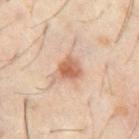{"biopsy_status": "not biopsied; imaged during a skin examination", "lighting": "cross-polarized", "lesion_size": {"long_diameter_mm_approx": 3.0}, "site": "abdomen", "automated_metrics": {"area_mm2_approx": 5.5, "eccentricity": 0.4, "shape_asymmetry": 0.25, "color_variation_0_10": 4.0, "peripheral_color_asymmetry": 1.0}, "patient": {"sex": "male", "age_approx": 60}, "image": {"source": "total-body photography crop", "field_of_view_mm": 15}}